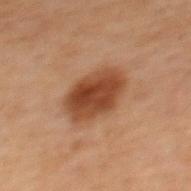{
  "biopsy_status": "not biopsied; imaged during a skin examination",
  "patient": {
    "sex": "male",
    "age_approx": 70
  },
  "image": {
    "source": "total-body photography crop",
    "field_of_view_mm": 15
  },
  "automated_metrics": {
    "eccentricity": 0.75,
    "shape_asymmetry": 0.1,
    "cielab_L": 33,
    "cielab_a": 19,
    "cielab_b": 27,
    "vs_skin_darker_L": 11.0,
    "vs_skin_contrast_norm": 10.0
  },
  "lesion_size": {
    "long_diameter_mm_approx": 5.0
  },
  "site": "mid back"
}